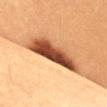Case summary:
– follow-up: imaged on a skin check; not biopsied
– patient: female, aged 28 to 32
– body site: the chest
– illumination: cross-polarized illumination
– imaging modality: ~15 mm tile from a whole-body skin photo
– lesion diameter: ~8 mm (longest diameter)
– automated metrics: two-axis asymmetry of about 0.3; roughly 23 lightness units darker than nearby skin and a normalized lesion–skin contrast near 15.5; a border-irregularity index near 4/10, internal color variation of about 7.5 on a 0–10 scale, and a peripheral color-asymmetry measure near 2.5; a nevus-likeness score of about 100/100